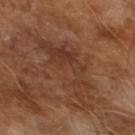Imaged during a routine full-body skin examination; the lesion was not biopsied and no histopathology is available.
A close-up tile cropped from a whole-body skin photograph, about 15 mm across.
The patient is a male aged around 65.
This is a cross-polarized tile.
The total-body-photography lesion software estimated a footprint of about 20 mm² and two-axis asymmetry of about 0.55. The analysis additionally found roughly 6 lightness units darker than nearby skin and a normalized border contrast of about 6. It also reported a color-variation rating of about 4.5/10 and peripheral color asymmetry of about 1.5.